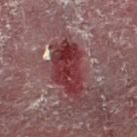biopsy status=imaged on a skin check; not biopsied
diameter=about 7 mm
subject=male, about 55 years old
tile lighting=cross-polarized illumination
automated lesion analysis=a shape eccentricity near 0.85; internal color variation of about 5 on a 0–10 scale and a peripheral color-asymmetry measure near 1.5
body site=the right lower leg
image=15 mm crop, total-body photography